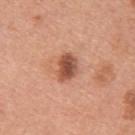Impression: The lesion was photographed on a routine skin check and not biopsied; there is no pathology result. Context: A lesion tile, about 15 mm wide, cut from a 3D total-body photograph. The tile uses white-light illumination. A male patient, aged 28–32. From the upper back.